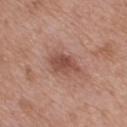The lesion was photographed on a routine skin check and not biopsied; there is no pathology result. The lesion-visualizer software estimated a lesion area of about 7.5 mm², a shape eccentricity near 0.8, and a shape-asymmetry score of about 0.2 (0 = symmetric). And it measured roughly 11 lightness units darker than nearby skin and a lesion-to-skin contrast of about 8 (normalized; higher = more distinct). And it measured a classifier nevus-likeness of about 70/100 and lesion-presence confidence of about 100/100. A 15 mm close-up extracted from a 3D total-body photography capture. The lesion is on the upper back. A male subject in their mid- to late 60s. Measured at roughly 4 mm in maximum diameter. The tile uses white-light illumination.